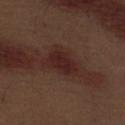<tbp_lesion>
  <automated_metrics>
    <vs_skin_darker_L>7.0</vs_skin_darker_L>
    <vs_skin_contrast_norm>9.0</vs_skin_contrast_norm>
    <nevus_likeness_0_100>20</nevus_likeness_0_100>
  </automated_metrics>
  <lighting>white-light</lighting>
  <site>front of the torso</site>
  <patient>
    <sex>male</sex>
    <age_approx>70</age_approx>
  </patient>
  <image>
    <source>total-body photography crop</source>
    <field_of_view_mm>15</field_of_view_mm>
  </image>
</tbp_lesion>